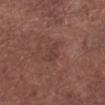No biopsy was performed on this lesion — it was imaged during a full skin examination and was not determined to be concerning. This is a white-light tile. A male subject, approximately 55 years of age. Longest diameter approximately 2.5 mm. A lesion tile, about 15 mm wide, cut from a 3D total-body photograph. The lesion is located on the left lower leg.